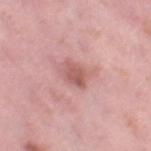Impression:
Part of a total-body skin-imaging series; this lesion was reviewed on a skin check and was not flagged for biopsy.
Context:
Approximately 2.5 mm at its widest. Imaged with white-light lighting. A female patient, aged 38–42. On the right thigh. This image is a 15 mm lesion crop taken from a total-body photograph.